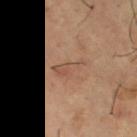Q: Was this lesion biopsied?
A: no biopsy performed (imaged during a skin exam)
Q: Automated lesion metrics?
A: a lesion area of about 5 mm², an eccentricity of roughly 0.9, and a shape-asymmetry score of about 0.4 (0 = symmetric); a lesion color around L≈51 a*≈20 b*≈29 in CIELAB and a normalized lesion–skin contrast near 5; a within-lesion color-variation index near 2/10 and radial color variation of about 0.5; a classifier nevus-likeness of about 0/100 and a detector confidence of about 100 out of 100 that the crop contains a lesion
Q: What is the imaging modality?
A: 15 mm crop, total-body photography
Q: Patient demographics?
A: male, aged approximately 65
Q: What is the anatomic site?
A: the leg
Q: What is the lesion's diameter?
A: ≈4 mm
Q: How was the tile lit?
A: cross-polarized illumination